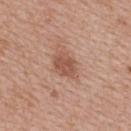Imaged during a routine full-body skin examination; the lesion was not biopsied and no histopathology is available.
A female subject, approximately 50 years of age.
Located on the upper back.
This image is a 15 mm lesion crop taken from a total-body photograph.